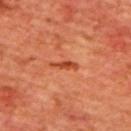biopsy status: catalogued during a skin exam; not biopsied | site: the back | subject: male, aged approximately 65 | image source: total-body-photography crop, ~15 mm field of view | diameter: about 3.5 mm | automated lesion analysis: an average lesion color of about L≈43 a*≈31 b*≈37 (CIELAB); an automated nevus-likeness rating near 5 out of 100 and lesion-presence confidence of about 95/100 | lighting: cross-polarized.